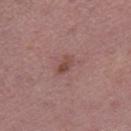Assessment: Captured during whole-body skin photography for melanoma surveillance; the lesion was not biopsied. Image and clinical context: Captured under white-light illumination. The patient is a male aged 38–42. The lesion is on the right thigh. Approximately 2.5 mm at its widest. A 15 mm crop from a total-body photograph taken for skin-cancer surveillance.